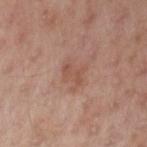Captured during whole-body skin photography for melanoma surveillance; the lesion was not biopsied.
The lesion is located on the left upper arm.
The recorded lesion diameter is about 3 mm.
Cropped from a total-body skin-imaging series; the visible field is about 15 mm.
Imaged with cross-polarized lighting.
A female patient, aged approximately 55.